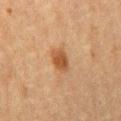<tbp_lesion>
<patient>
  <sex>male</sex>
  <age_approx>75</age_approx>
</patient>
<image>
  <source>total-body photography crop</source>
  <field_of_view_mm>15</field_of_view_mm>
</image>
<automated_metrics>
  <area_mm2_approx>5.0</area_mm2_approx>
  <eccentricity>0.85</eccentricity>
  <shape_asymmetry>0.15</shape_asymmetry>
  <border_irregularity_0_10>2.0</border_irregularity_0_10>
  <color_variation_0_10>2.0</color_variation_0_10>
  <peripheral_color_asymmetry>0.5</peripheral_color_asymmetry>
</automated_metrics>
<site>chest</site>
</tbp_lesion>